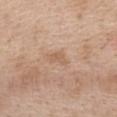Impression: Imaged during a routine full-body skin examination; the lesion was not biopsied and no histopathology is available. Clinical summary: A lesion tile, about 15 mm wide, cut from a 3D total-body photograph. A female subject, aged approximately 40. Automated tile analysis of the lesion measured border irregularity of about 4 on a 0–10 scale, a color-variation rating of about 1/10, and peripheral color asymmetry of about 0.5. The lesion is on the chest. Approximately 3 mm at its widest. Captured under white-light illumination.